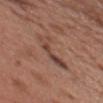workup: total-body-photography surveillance lesion; no biopsy
image-analysis metrics: a footprint of about 7.5 mm² and a shape-asymmetry score of about 0.5 (0 = symmetric); a lesion color around L≈44 a*≈20 b*≈26 in CIELAB; an automated nevus-likeness rating near 0 out of 100 and lesion-presence confidence of about 70/100
subject: male, in their mid- to late 50s
location: the chest
lighting: white-light illumination
size: ≈5.5 mm
image: ~15 mm tile from a whole-body skin photo